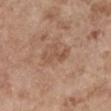Captured during whole-body skin photography for melanoma surveillance; the lesion was not biopsied. Cropped from a whole-body photographic skin survey; the tile spans about 15 mm. The total-body-photography lesion software estimated an area of roughly 4 mm², a shape eccentricity near 0.95, and two-axis asymmetry of about 0.45. The software also gave an average lesion color of about L≈52 a*≈20 b*≈30 (CIELAB), a lesion–skin lightness drop of about 7, and a lesion-to-skin contrast of about 5.5 (normalized; higher = more distinct). And it measured a border-irregularity index near 6/10, internal color variation of about 0 on a 0–10 scale, and a peripheral color-asymmetry measure near 0. Longest diameter approximately 3.5 mm. The tile uses white-light illumination. On the right upper arm. A female patient in their mid- to late 60s.